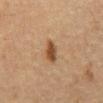biopsy_status: not biopsied; imaged during a skin examination
lesion_size:
  long_diameter_mm_approx: 3.5
site: front of the torso
lighting: cross-polarized
image:
  source: total-body photography crop
  field_of_view_mm: 15
patient:
  sex: male
  age_approx: 65